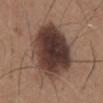This lesion was catalogued during total-body skin photography and was not selected for biopsy.